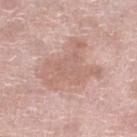Captured during whole-body skin photography for melanoma surveillance; the lesion was not biopsied. The subject is a female aged approximately 60. This image is a 15 mm lesion crop taken from a total-body photograph. On the leg. Captured under white-light illumination. The lesion's longest dimension is about 6.5 mm.